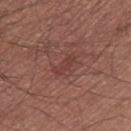No biopsy was performed on this lesion — it was imaged during a full skin examination and was not determined to be concerning.
A region of skin cropped from a whole-body photographic capture, roughly 15 mm wide.
The lesion is on the right thigh.
A male subject, aged approximately 55.